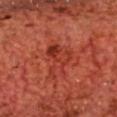The lesion was photographed on a routine skin check and not biopsied; there is no pathology result.
A 15 mm close-up extracted from a 3D total-body photography capture.
On the chest.
The patient is a male roughly 70 years of age.